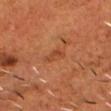Assessment: This lesion was catalogued during total-body skin photography and was not selected for biopsy. Context: A lesion tile, about 15 mm wide, cut from a 3D total-body photograph. The lesion is located on the head or neck. An algorithmic analysis of the crop reported an area of roughly 3 mm², an outline eccentricity of about 0.85 (0 = round, 1 = elongated), and a symmetry-axis asymmetry near 0.4. And it measured roughly 6 lightness units darker than nearby skin. And it measured a classifier nevus-likeness of about 0/100 and a detector confidence of about 100 out of 100 that the crop contains a lesion. A male subject aged approximately 55.